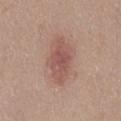notes — imaged on a skin check; not biopsied | tile lighting — white-light illumination | patient — female, approximately 40 years of age | site — the mid back | diameter — ≈5.5 mm | image source — total-body-photography crop, ~15 mm field of view.